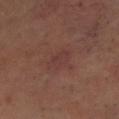No biopsy was performed on this lesion — it was imaged during a full skin examination and was not determined to be concerning. Longest diameter approximately 3 mm. On the head or neck. The subject is a male aged 63 to 67. The lesion-visualizer software estimated a lesion area of about 6 mm², an eccentricity of roughly 0.65, and a shape-asymmetry score of about 0.25 (0 = symmetric). It also reported a mean CIELAB color near L≈35 a*≈20 b*≈21, a lesion–skin lightness drop of about 4, and a normalized border contrast of about 4.5. The analysis additionally found a classifier nevus-likeness of about 5/100 and a lesion-detection confidence of about 100/100. This image is a 15 mm lesion crop taken from a total-body photograph.